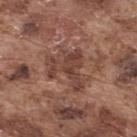biopsy_status: not biopsied; imaged during a skin examination
site: upper back
image:
  source: total-body photography crop
  field_of_view_mm: 15
patient:
  sex: male
  age_approx: 75
lighting: white-light
automated_metrics:
  cielab_L: 42
  cielab_a: 21
  cielab_b: 25
  vs_skin_darker_L: 9.0
  vs_skin_contrast_norm: 7.0
  border_irregularity_0_10: 7.0
  color_variation_0_10: 4.5
  peripheral_color_asymmetry: 1.5